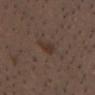Clinical impression: The lesion was photographed on a routine skin check and not biopsied; there is no pathology result. Clinical summary: Approximately 3 mm at its widest. A 15 mm crop from a total-body photograph taken for skin-cancer surveillance. The subject is a male aged approximately 40. Located on the head or neck. Imaged with white-light lighting.